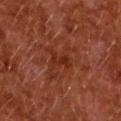Q: Was this lesion biopsied?
A: total-body-photography surveillance lesion; no biopsy
Q: How was this image acquired?
A: ~15 mm tile from a whole-body skin photo
Q: How large is the lesion?
A: ≈3 mm
Q: What is the anatomic site?
A: the upper back
Q: How was the tile lit?
A: cross-polarized
Q: What are the patient's age and sex?
A: female, approximately 50 years of age
Q: Automated lesion metrics?
A: a lesion area of about 4 mm², a shape eccentricity near 0.8, and a symmetry-axis asymmetry near 0.5; an average lesion color of about L≈23 a*≈23 b*≈26 (CIELAB), roughly 5 lightness units darker than nearby skin, and a normalized lesion–skin contrast near 6; a border-irregularity rating of about 5.5/10, internal color variation of about 2 on a 0–10 scale, and a peripheral color-asymmetry measure near 0.5; a detector confidence of about 100 out of 100 that the crop contains a lesion